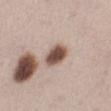<tbp_lesion>
  <biopsy_status>not biopsied; imaged during a skin examination</biopsy_status>
  <site>left lower leg</site>
  <lighting>white-light</lighting>
  <image>
    <source>total-body photography crop</source>
    <field_of_view_mm>15</field_of_view_mm>
  </image>
  <lesion_size>
    <long_diameter_mm_approx>3.5</long_diameter_mm_approx>
  </lesion_size>
  <patient>
    <sex>female</sex>
    <age_approx>40</age_approx>
  </patient>
</tbp_lesion>